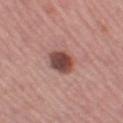biopsy status = no biopsy performed (imaged during a skin exam).